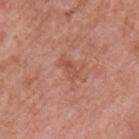Impression:
No biopsy was performed on this lesion — it was imaged during a full skin examination and was not determined to be concerning.
Background:
The lesion is located on the left upper arm. A male patient, aged approximately 70. About 3 mm across. An algorithmic analysis of the crop reported a lesion area of about 3.5 mm², an eccentricity of roughly 0.9, and a shape-asymmetry score of about 0.4 (0 = symmetric). The analysis additionally found a mean CIELAB color near L≈52 a*≈26 b*≈30, roughly 7 lightness units darker than nearby skin, and a normalized lesion–skin contrast near 5. The software also gave a nevus-likeness score of about 0/100 and a detector confidence of about 100 out of 100 that the crop contains a lesion. Cropped from a total-body skin-imaging series; the visible field is about 15 mm. Imaged with white-light lighting.